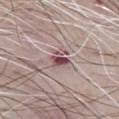Findings:
* biopsy status: total-body-photography surveillance lesion; no biopsy